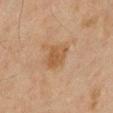Assessment: The lesion was tiled from a total-body skin photograph and was not biopsied. Background: Measured at roughly 3 mm in maximum diameter. Located on the mid back. The patient is a male in their mid- to late 40s. The tile uses cross-polarized illumination. The total-body-photography lesion software estimated a mean CIELAB color near L≈43 a*≈16 b*≈30 and a lesion-to-skin contrast of about 6.5 (normalized; higher = more distinct). The software also gave border irregularity of about 2.5 on a 0–10 scale, a within-lesion color-variation index near 2/10, and peripheral color asymmetry of about 0.5. The analysis additionally found an automated nevus-likeness rating near 0 out of 100. A lesion tile, about 15 mm wide, cut from a 3D total-body photograph.